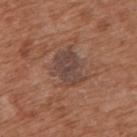* subject: female, roughly 75 years of age
* automated metrics: an area of roughly 13 mm² and an outline eccentricity of about 0.6 (0 = round, 1 = elongated); an average lesion color of about L≈43 a*≈18 b*≈23 (CIELAB), a lesion–skin lightness drop of about 8, and a normalized lesion–skin contrast near 7.5
* image: total-body-photography crop, ~15 mm field of view
* illumination: white-light
* anatomic site: the upper back